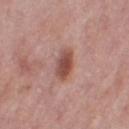biopsy status: total-body-photography surveillance lesion; no biopsy
subject: female, aged 48 to 52
image source: 15 mm crop, total-body photography
body site: the right thigh